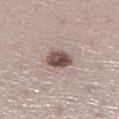Impression:
The lesion was tiled from a total-body skin photograph and was not biopsied.
Clinical summary:
The tile uses white-light illumination. On the right lower leg. The subject is a male approximately 60 years of age. A lesion tile, about 15 mm wide, cut from a 3D total-body photograph. Longest diameter approximately 3 mm.